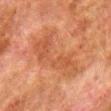Notes:
– notes · imaged on a skin check; not biopsied
– body site · the right lower leg
– automated metrics · a nevus-likeness score of about 0/100 and a lesion-detection confidence of about 100/100
– image source · ~15 mm crop, total-body skin-cancer survey
– tile lighting · cross-polarized illumination
– patient · male, approximately 80 years of age